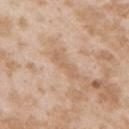A female subject aged 23 to 27.
The lesion is on the right upper arm.
The tile uses white-light illumination.
A roughly 15 mm field-of-view crop from a total-body skin photograph.
The total-body-photography lesion software estimated an outline eccentricity of about 0.95 (0 = round, 1 = elongated) and two-axis asymmetry of about 0.4. The software also gave a lesion color around L≈62 a*≈18 b*≈33 in CIELAB, roughly 6 lightness units darker than nearby skin, and a normalized border contrast of about 5.
About 4 mm across.